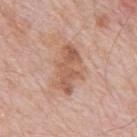Q: Was this lesion biopsied?
A: imaged on a skin check; not biopsied
Q: Where on the body is the lesion?
A: the mid back
Q: How was this image acquired?
A: ~15 mm tile from a whole-body skin photo
Q: What is the lesion's diameter?
A: ~6 mm (longest diameter)
Q: How was the tile lit?
A: white-light illumination
Q: Who is the patient?
A: male, aged 78 to 82